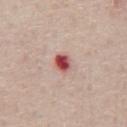notes: imaged on a skin check; not biopsied
subject: male, in their 60s
acquisition: ~15 mm tile from a whole-body skin photo
anatomic site: the front of the torso
tile lighting: white-light
lesion diameter: ~2.5 mm (longest diameter)
automated metrics: an area of roughly 4 mm², an eccentricity of roughly 0.65, and a shape-asymmetry score of about 0.15 (0 = symmetric); a mean CIELAB color near L≈52 a*≈33 b*≈24, a lesion–skin lightness drop of about 18, and a normalized lesion–skin contrast near 11.5; a border-irregularity index near 1.5/10, a color-variation rating of about 6/10, and radial color variation of about 2